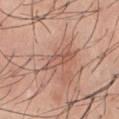Assessment: This lesion was catalogued during total-body skin photography and was not selected for biopsy. Acquisition and patient details: Automated image analysis of the tile measured a detector confidence of about 65 out of 100 that the crop contains a lesion. The subject is a male approximately 45 years of age. On the chest. A 15 mm crop from a total-body photograph taken for skin-cancer surveillance. Imaged with white-light lighting. The lesion's longest dimension is about 4.5 mm.